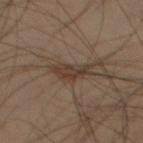Assessment:
The lesion was photographed on a routine skin check and not biopsied; there is no pathology result.
Context:
The patient is a male aged 38–42. From the left thigh. A lesion tile, about 15 mm wide, cut from a 3D total-body photograph.